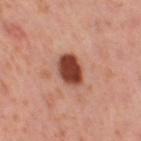Imaged during a routine full-body skin examination; the lesion was not biopsied and no histopathology is available.
The subject is a female in their 40s.
The tile uses cross-polarized illumination.
From the leg.
This image is a 15 mm lesion crop taken from a total-body photograph.